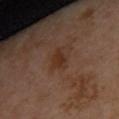Q: Was this lesion biopsied?
A: total-body-photography surveillance lesion; no biopsy
Q: What kind of image is this?
A: ~15 mm tile from a whole-body skin photo
Q: What are the patient's age and sex?
A: female, in their 40s
Q: What is the lesion's diameter?
A: ≈3 mm
Q: What is the anatomic site?
A: the back